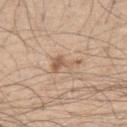{
  "biopsy_status": "not biopsied; imaged during a skin examination",
  "lighting": "white-light",
  "site": "right upper arm",
  "patient": {
    "sex": "male",
    "age_approx": 30
  },
  "image": {
    "source": "total-body photography crop",
    "field_of_view_mm": 15
  }
}